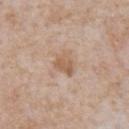<record>
<biopsy_status>not biopsied; imaged during a skin examination</biopsy_status>
<image>
  <source>total-body photography crop</source>
  <field_of_view_mm>15</field_of_view_mm>
</image>
<site>chest</site>
<patient>
  <sex>male</sex>
  <age_approx>65</age_approx>
</patient>
</record>